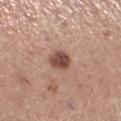Assessment: No biopsy was performed on this lesion — it was imaged during a full skin examination and was not determined to be concerning. Image and clinical context: The subject is a female aged 58 to 62. From the left lower leg. Longest diameter approximately 3 mm. This is a white-light tile. This image is a 15 mm lesion crop taken from a total-body photograph.